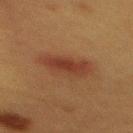Recorded during total-body skin imaging; not selected for excision or biopsy. The lesion-visualizer software estimated a border-irregularity rating of about 2.5/10, a within-lesion color-variation index near 4/10, and radial color variation of about 1. The analysis additionally found a classifier nevus-likeness of about 95/100 and a detector confidence of about 100 out of 100 that the crop contains a lesion. Captured under cross-polarized illumination. The lesion's longest dimension is about 5.5 mm. On the back. A female patient, aged approximately 40. This image is a 15 mm lesion crop taken from a total-body photograph.